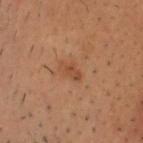| field | value |
|---|---|
| notes | imaged on a skin check; not biopsied |
| anatomic site | the head or neck |
| tile lighting | cross-polarized illumination |
| patient | male, roughly 30 years of age |
| imaging modality | total-body-photography crop, ~15 mm field of view |
| automated lesion analysis | a lesion area of about 3 mm², an outline eccentricity of about 0.8 (0 = round, 1 = elongated), and two-axis asymmetry of about 0.25; a border-irregularity index near 2.5/10 and a peripheral color-asymmetry measure near 0 |
| lesion diameter | about 2.5 mm |